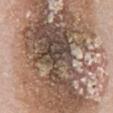Q: Was a biopsy performed?
A: imaged on a skin check; not biopsied
Q: How was the tile lit?
A: white-light illumination
Q: How was this image acquired?
A: ~15 mm crop, total-body skin-cancer survey
Q: What is the anatomic site?
A: the abdomen
Q: Patient demographics?
A: male, approximately 80 years of age